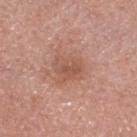Imaged during a routine full-body skin examination; the lesion was not biopsied and no histopathology is available. This is a white-light tile. The patient is a male about 65 years old. The recorded lesion diameter is about 3.5 mm. A region of skin cropped from a whole-body photographic capture, roughly 15 mm wide. On the head or neck.